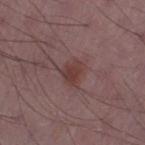biopsy status — no biopsy performed (imaged during a skin exam) | image — ~15 mm tile from a whole-body skin photo | illumination — white-light | anatomic site — the left thigh | size — about 2.5 mm | patient — male, roughly 50 years of age | TBP lesion metrics — border irregularity of about 3 on a 0–10 scale, internal color variation of about 2 on a 0–10 scale, and radial color variation of about 0.5.